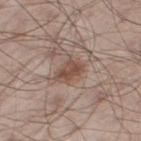No biopsy was performed on this lesion — it was imaged during a full skin examination and was not determined to be concerning. Cropped from a whole-body photographic skin survey; the tile spans about 15 mm. A male patient aged around 55. The lesion is located on the right thigh.